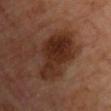Imaged with cross-polarized lighting.
Longest diameter approximately 7 mm.
On the chest.
A male subject, about 60 years old.
A close-up tile cropped from a whole-body skin photograph, about 15 mm across.